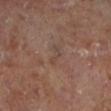notes — total-body-photography surveillance lesion; no biopsy
lesion diameter — ≈3 mm
location — the left lower leg
automated lesion analysis — a footprint of about 2.5 mm² and a shape eccentricity near 0.9; a border-irregularity rating of about 6/10, a color-variation rating of about 0/10, and a peripheral color-asymmetry measure near 0; a nevus-likeness score of about 0/100 and lesion-presence confidence of about 100/100
tile lighting — cross-polarized illumination
image — ~15 mm crop, total-body skin-cancer survey